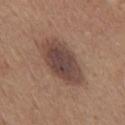The lesion was tiled from a total-body skin photograph and was not biopsied. On the chest. Measured at roughly 6.5 mm in maximum diameter. Imaged with white-light lighting. Automated tile analysis of the lesion measured a footprint of about 17 mm², an eccentricity of roughly 0.85, and two-axis asymmetry of about 0.15. It also reported an average lesion color of about L≈43 a*≈17 b*≈22 (CIELAB) and a lesion–skin lightness drop of about 12. A 15 mm close-up tile from a total-body photography series done for melanoma screening. The subject is a male approximately 65 years of age.